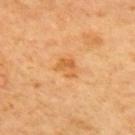  biopsy_status: not biopsied; imaged during a skin examination
  image:
    source: total-body photography crop
    field_of_view_mm: 15
  patient:
    sex: male
    age_approx: 65
  site: mid back